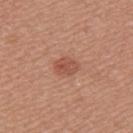{"biopsy_status": "not biopsied; imaged during a skin examination"}